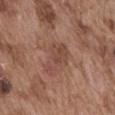Part of a total-body skin-imaging series; this lesion was reviewed on a skin check and was not flagged for biopsy.
This image is a 15 mm lesion crop taken from a total-body photograph.
The total-body-photography lesion software estimated a footprint of about 9 mm², an eccentricity of roughly 0.8, and two-axis asymmetry of about 0.45. The software also gave a lesion color around L≈46 a*≈21 b*≈26 in CIELAB, roughly 7 lightness units darker than nearby skin, and a normalized lesion–skin contrast near 5.5. And it measured border irregularity of about 5.5 on a 0–10 scale and a color-variation rating of about 2.5/10.
The recorded lesion diameter is about 4.5 mm.
The subject is a male approximately 75 years of age.
Located on the abdomen.